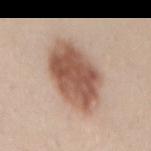  biopsy_status: not biopsied; imaged during a skin examination
  image:
    source: total-body photography crop
    field_of_view_mm: 15
  lesion_size:
    long_diameter_mm_approx: 7.5
  patient:
    sex: female
    age_approx: 25
  lighting: white-light
  site: back